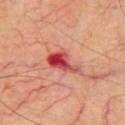No biopsy was performed on this lesion — it was imaged during a full skin examination and was not determined to be concerning. The recorded lesion diameter is about 4.5 mm. The total-body-photography lesion software estimated an outline eccentricity of about 0.85 (0 = round, 1 = elongated) and two-axis asymmetry of about 0.4. It also reported border irregularity of about 4.5 on a 0–10 scale and internal color variation of about 9.5 on a 0–10 scale. The lesion is located on the chest. Imaged with cross-polarized lighting. A male subject, aged 68–72. A roughly 15 mm field-of-view crop from a total-body skin photograph.